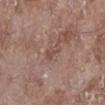biopsy status: imaged on a skin check; not biopsied
patient: female, aged around 75
location: the leg
illumination: white-light illumination
image source: total-body-photography crop, ~15 mm field of view